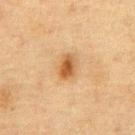Q: Automated lesion metrics?
A: a border-irregularity index near 1.5/10, internal color variation of about 4 on a 0–10 scale, and a peripheral color-asymmetry measure near 1
Q: What kind of image is this?
A: 15 mm crop, total-body photography
Q: Illumination type?
A: cross-polarized
Q: What is the lesion's diameter?
A: ~3 mm (longest diameter)
Q: What is the anatomic site?
A: the front of the torso
Q: What are the patient's age and sex?
A: male, about 75 years old